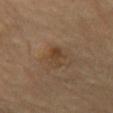Imaged during a routine full-body skin examination; the lesion was not biopsied and no histopathology is available. A region of skin cropped from a whole-body photographic capture, roughly 15 mm wide. Imaged with cross-polarized lighting. On the chest. A male subject, aged approximately 85 or older.